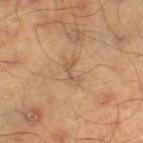Impression: The lesion was photographed on a routine skin check and not biopsied; there is no pathology result. Context: This image is a 15 mm lesion crop taken from a total-body photograph. Measured at roughly 3.5 mm in maximum diameter. From the left thigh. A male patient, aged approximately 45. The lesion-visualizer software estimated border irregularity of about 6.5 on a 0–10 scale and peripheral color asymmetry of about 0. Captured under cross-polarized illumination.